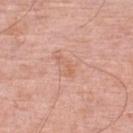{"biopsy_status": "not biopsied; imaged during a skin examination", "lesion_size": {"long_diameter_mm_approx": 3.0}, "patient": {"sex": "male", "age_approx": 80}, "image": {"source": "total-body photography crop", "field_of_view_mm": 15}, "site": "left lower leg", "automated_metrics": {"cielab_L": 63, "cielab_a": 23, "cielab_b": 31, "vs_skin_darker_L": 6.0, "vs_skin_contrast_norm": 5.0, "border_irregularity_0_10": 6.0, "nevus_likeness_0_100": 0, "lesion_detection_confidence_0_100": 100}}